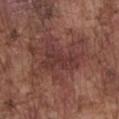Case summary:
- follow-up · total-body-photography surveillance lesion; no biopsy
- subject · male, roughly 75 years of age
- lesion size · about 4.5 mm
- site · the chest
- tile lighting · white-light
- image · 15 mm crop, total-body photography